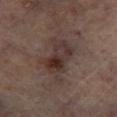Captured during whole-body skin photography for melanoma surveillance; the lesion was not biopsied.
The lesion is located on the left lower leg.
A male subject aged around 65.
A 15 mm crop from a total-body photograph taken for skin-cancer surveillance.